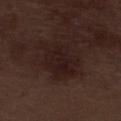No biopsy was performed on this lesion — it was imaged during a full skin examination and was not determined to be concerning. A male patient about 70 years old. About 5 mm across. A 15 mm close-up extracted from a 3D total-body photography capture. On the left lower leg. The lesion-visualizer software estimated a border-irregularity rating of about 2.5/10, a within-lesion color-variation index near 3/10, and radial color variation of about 1. The analysis additionally found a nevus-likeness score of about 0/100 and a detector confidence of about 100 out of 100 that the crop contains a lesion. The tile uses white-light illumination.